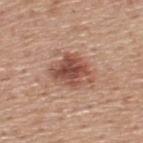Part of a total-body skin-imaging series; this lesion was reviewed on a skin check and was not flagged for biopsy. A 15 mm close-up tile from a total-body photography series done for melanoma screening. From the upper back. Longest diameter approximately 5 mm. Captured under white-light illumination. A male patient aged 58 to 62.